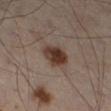Recorded during total-body skin imaging; not selected for excision or biopsy.
A close-up tile cropped from a whole-body skin photograph, about 15 mm across.
Imaged with cross-polarized lighting.
A male patient in their 50s.
From the left leg.
About 3.5 mm across.